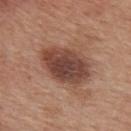Case summary:
• follow-up — total-body-photography surveillance lesion; no biopsy
• subject — male, aged around 30
• image — 15 mm crop, total-body photography
• size — ≈6 mm
• image-analysis metrics — border irregularity of about 1.5 on a 0–10 scale; a classifier nevus-likeness of about 45/100 and a lesion-detection confidence of about 100/100
• anatomic site — the upper back